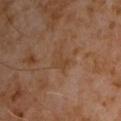Clinical impression: No biopsy was performed on this lesion — it was imaged during a full skin examination and was not determined to be concerning. Context: The lesion is on the chest. A male patient aged 58 to 62. The tile uses cross-polarized illumination. About 2.5 mm across. A close-up tile cropped from a whole-body skin photograph, about 15 mm across. An algorithmic analysis of the crop reported a lesion area of about 4 mm², an outline eccentricity of about 0.7 (0 = round, 1 = elongated), and a shape-asymmetry score of about 0.45 (0 = symmetric). And it measured a border-irregularity index near 5/10, a within-lesion color-variation index near 0.5/10, and peripheral color asymmetry of about 0.